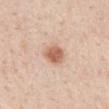- patient: female, aged around 45
- automated lesion analysis: a footprint of about 6 mm² and an outline eccentricity of about 0.65 (0 = round, 1 = elongated); a border-irregularity index near 2/10
- image: total-body-photography crop, ~15 mm field of view
- body site: the back
- tile lighting: white-light
- diameter: about 3 mm